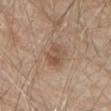Assessment:
Captured during whole-body skin photography for melanoma surveillance; the lesion was not biopsied.
Acquisition and patient details:
A male patient, roughly 80 years of age. A 15 mm close-up extracted from a 3D total-body photography capture. Imaged with white-light lighting. The total-body-photography lesion software estimated a footprint of about 6.5 mm², an eccentricity of roughly 0.5, and a shape-asymmetry score of about 0.2 (0 = symmetric). The analysis additionally found a border-irregularity index near 2/10, a color-variation rating of about 4/10, and peripheral color asymmetry of about 1.5. The analysis additionally found a classifier nevus-likeness of about 40/100. Located on the front of the torso.